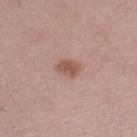biopsy status: catalogued during a skin exam; not biopsied
imaging modality: ~15 mm tile from a whole-body skin photo
subject: female, about 45 years old
image-analysis metrics: an average lesion color of about L≈53 a*≈22 b*≈25 (CIELAB), roughly 11 lightness units darker than nearby skin, and a normalized border contrast of about 8; border irregularity of about 1.5 on a 0–10 scale, a color-variation rating of about 1.5/10, and a peripheral color-asymmetry measure near 0.5; a classifier nevus-likeness of about 90/100
size: about 2.5 mm
anatomic site: the leg
tile lighting: white-light